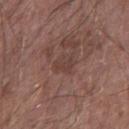No biopsy was performed on this lesion — it was imaged during a full skin examination and was not determined to be concerning. A lesion tile, about 15 mm wide, cut from a 3D total-body photograph. The lesion's longest dimension is about 2.5 mm. The patient is a male roughly 65 years of age. The tile uses white-light illumination. The lesion is located on the right forearm.